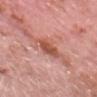The lesion was tiled from a total-body skin photograph and was not biopsied.
The total-body-photography lesion software estimated a lesion color around L≈54 a*≈28 b*≈30 in CIELAB, a lesion–skin lightness drop of about 11, and a normalized lesion–skin contrast near 8.
The patient is a male in their 80s.
On the head or neck.
A close-up tile cropped from a whole-body skin photograph, about 15 mm across.